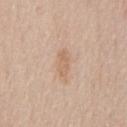Captured during whole-body skin photography for melanoma surveillance; the lesion was not biopsied. A roughly 15 mm field-of-view crop from a total-body skin photograph. The subject is a male approximately 65 years of age. About 3 mm across. Automated tile analysis of the lesion measured a border-irregularity index near 4/10, a within-lesion color-variation index near 0/10, and radial color variation of about 0. Located on the chest.